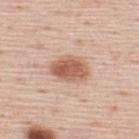Q: Was this lesion biopsied?
A: catalogued during a skin exam; not biopsied
Q: Patient demographics?
A: male, aged approximately 45
Q: What is the imaging modality?
A: ~15 mm tile from a whole-body skin photo
Q: What is the anatomic site?
A: the upper back